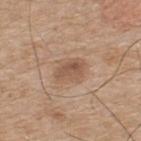notes = no biopsy performed (imaged during a skin exam) | subject = male, about 75 years old | location = the upper back | acquisition = ~15 mm crop, total-body skin-cancer survey | lesion size = ~3.5 mm (longest diameter) | illumination = white-light illumination | image-analysis metrics = a normalized border contrast of about 6.5; border irregularity of about 2 on a 0–10 scale, internal color variation of about 4.5 on a 0–10 scale, and peripheral color asymmetry of about 1.5.